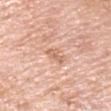<case>
  <biopsy_status>not biopsied; imaged during a skin examination</biopsy_status>
  <lesion_size>
    <long_diameter_mm_approx>2.5</long_diameter_mm_approx>
  </lesion_size>
  <site>right upper arm</site>
  <patient>
    <sex>male</sex>
    <age_approx>60</age_approx>
  </patient>
  <image>
    <source>total-body photography crop</source>
    <field_of_view_mm>15</field_of_view_mm>
  </image>
  <lighting>white-light</lighting>
</case>